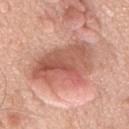biopsy status: total-body-photography surveillance lesion; no biopsy | illumination: white-light | image source: ~15 mm tile from a whole-body skin photo | body site: the mid back | patient: male, in their mid-70s | image-analysis metrics: a mean CIELAB color near L≈58 a*≈26 b*≈29, a lesion–skin lightness drop of about 13, and a lesion-to-skin contrast of about 8 (normalized; higher = more distinct); an automated nevus-likeness rating near 55 out of 100 | lesion diameter: ≈8.5 mm.